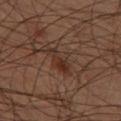Assessment:
Part of a total-body skin-imaging series; this lesion was reviewed on a skin check and was not flagged for biopsy.
Clinical summary:
A male patient aged 58 to 62. A 15 mm crop from a total-body photograph taken for skin-cancer surveillance. The lesion is located on the right thigh.